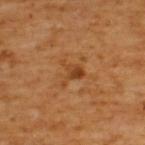Captured during whole-body skin photography for melanoma surveillance; the lesion was not biopsied. The total-body-photography lesion software estimated an area of roughly 4.5 mm² and an outline eccentricity of about 0.4 (0 = round, 1 = elongated). The software also gave a lesion–skin lightness drop of about 8. And it measured a border-irregularity rating of about 7/10 and a color-variation rating of about 4/10. A male patient aged around 65. A close-up tile cropped from a whole-body skin photograph, about 15 mm across. Imaged with cross-polarized lighting. The lesion is located on the upper back. Longest diameter approximately 3 mm.